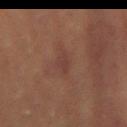{
  "biopsy_status": "not biopsied; imaged during a skin examination",
  "lesion_size": {
    "long_diameter_mm_approx": 3.5
  },
  "image": {
    "source": "total-body photography crop",
    "field_of_view_mm": 15
  },
  "patient": {
    "sex": "female",
    "age_approx": 60
  },
  "site": "abdomen"
}